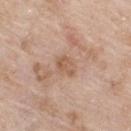Findings:
– follow-up: total-body-photography surveillance lesion; no biopsy
– illumination: white-light
– subject: female, aged around 75
– location: the right thigh
– automated metrics: a lesion color around L≈57 a*≈19 b*≈31 in CIELAB and a lesion–skin lightness drop of about 8; a border-irregularity index near 4/10 and a color-variation rating of about 2/10; lesion-presence confidence of about 100/100
– imaging modality: ~15 mm crop, total-body skin-cancer survey